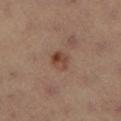The lesion was photographed on a routine skin check and not biopsied; there is no pathology result. An algorithmic analysis of the crop reported a lesion color around L≈43 a*≈20 b*≈28 in CIELAB, roughly 9 lightness units darker than nearby skin, and a normalized lesion–skin contrast near 7.5. It also reported border irregularity of about 1.5 on a 0–10 scale and a within-lesion color-variation index near 6.5/10. Captured under cross-polarized illumination. A female patient, aged around 35. The lesion is located on the left lower leg. The lesion's longest dimension is about 2.5 mm. A 15 mm close-up tile from a total-body photography series done for melanoma screening.